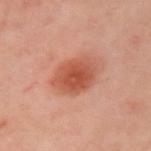image-analysis metrics — an area of roughly 14 mm² and a shape-asymmetry score of about 0.15 (0 = symmetric); border irregularity of about 1.5 on a 0–10 scale, a color-variation rating of about 4.5/10, and radial color variation of about 1; a classifier nevus-likeness of about 100/100 and lesion-presence confidence of about 100/100 | lesion size — about 5 mm | patient — female, in their mid-50s | acquisition — total-body-photography crop, ~15 mm field of view | body site — the arm.